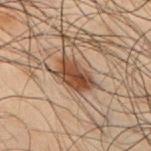Imaged during a routine full-body skin examination; the lesion was not biopsied and no histopathology is available.
A roughly 15 mm field-of-view crop from a total-body skin photograph.
From the back.
The lesion's longest dimension is about 4.5 mm.
A male patient, about 50 years old.